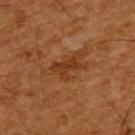Imaged during a routine full-body skin examination; the lesion was not biopsied and no histopathology is available.
About 4 mm across.
On the upper back.
Imaged with cross-polarized lighting.
The subject is a male aged 63 to 67.
The lesion-visualizer software estimated an area of roughly 5 mm² and an eccentricity of roughly 0.8. The analysis additionally found a lesion color around L≈29 a*≈21 b*≈30 in CIELAB.
A close-up tile cropped from a whole-body skin photograph, about 15 mm across.